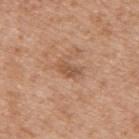Notes:
• workup — no biopsy performed (imaged during a skin exam)
• imaging modality — ~15 mm tile from a whole-body skin photo
• anatomic site — the upper back
• image-analysis metrics — an average lesion color of about L≈53 a*≈21 b*≈32 (CIELAB), a lesion–skin lightness drop of about 9, and a lesion-to-skin contrast of about 6.5 (normalized; higher = more distinct); a border-irregularity rating of about 2.5/10, a within-lesion color-variation index near 2/10, and a peripheral color-asymmetry measure near 0.5; a classifier nevus-likeness of about 5/100
• diameter — ≈3 mm
• lighting — white-light illumination
• patient — male, approximately 30 years of age